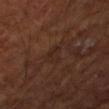The lesion was photographed on a routine skin check and not biopsied; there is no pathology result. A 15 mm crop from a total-body photograph taken for skin-cancer surveillance. The lesion is located on the left forearm. A male subject, approximately 65 years of age. Measured at roughly 2.5 mm in maximum diameter. Captured under cross-polarized illumination.